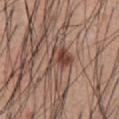{
  "biopsy_status": "not biopsied; imaged during a skin examination",
  "image": {
    "source": "total-body photography crop",
    "field_of_view_mm": 15
  },
  "lighting": "white-light",
  "automated_metrics": {
    "area_mm2_approx": 8.0,
    "eccentricity": 0.65,
    "cielab_L": 45,
    "cielab_a": 20,
    "cielab_b": 25,
    "vs_skin_darker_L": 11.0,
    "vs_skin_contrast_norm": 8.5
  },
  "patient": {
    "sex": "male",
    "age_approx": 55
  },
  "site": "abdomen"
}